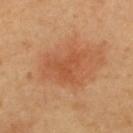Q: Was this lesion biopsied?
A: catalogued during a skin exam; not biopsied
Q: Lesion size?
A: about 5 mm
Q: What did automated image analysis measure?
A: a border-irregularity index near 6.5/10, a within-lesion color-variation index near 2/10, and radial color variation of about 0.5; a nevus-likeness score of about 50/100 and a detector confidence of about 100 out of 100 that the crop contains a lesion
Q: What is the imaging modality?
A: total-body-photography crop, ~15 mm field of view
Q: Who is the patient?
A: female, aged approximately 60
Q: What is the anatomic site?
A: the upper back
Q: How was the tile lit?
A: cross-polarized illumination